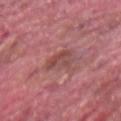biopsy status: no biopsy performed (imaged during a skin exam)
acquisition: 15 mm crop, total-body photography
site: the arm
patient: male, aged around 40